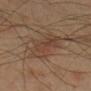biopsy_status: not biopsied; imaged during a skin examination
patient:
  sex: male
  age_approx: 70
lighting: cross-polarized
image:
  source: total-body photography crop
  field_of_view_mm: 15
site: right lower leg